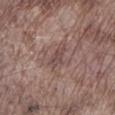Impression: This lesion was catalogued during total-body skin photography and was not selected for biopsy. Acquisition and patient details: Longest diameter approximately 3.5 mm. The lesion is on the left lower leg. The tile uses white-light illumination. A male subject aged approximately 75. The lesion-visualizer software estimated a lesion area of about 4 mm², an outline eccentricity of about 0.9 (0 = round, 1 = elongated), and a shape-asymmetry score of about 0.35 (0 = symmetric). It also reported an average lesion color of about L≈46 a*≈17 b*≈18 (CIELAB), roughly 8 lightness units darker than nearby skin, and a normalized border contrast of about 6.5. And it measured a border-irregularity index near 4/10. The software also gave a detector confidence of about 70 out of 100 that the crop contains a lesion. A roughly 15 mm field-of-view crop from a total-body skin photograph.